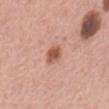Findings:
* workup: no biopsy performed (imaged during a skin exam)
* TBP lesion metrics: a mean CIELAB color near L≈55 a*≈25 b*≈29 and a normalized lesion–skin contrast near 8.5; a border-irregularity index near 1.5/10 and a color-variation rating of about 3/10; an automated nevus-likeness rating near 95 out of 100
* illumination: white-light
* body site: the front of the torso
* subject: female, in their mid-60s
* image: ~15 mm tile from a whole-body skin photo